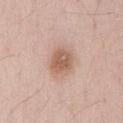| feature | finding |
|---|---|
| workup | catalogued during a skin exam; not biopsied |
| subject | male, aged 48 to 52 |
| diameter | ≈3 mm |
| location | the back |
| acquisition | total-body-photography crop, ~15 mm field of view |
| automated metrics | a nevus-likeness score of about 90/100 and a detector confidence of about 100 out of 100 that the crop contains a lesion |
| illumination | white-light |